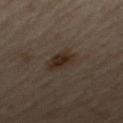<lesion>
  <biopsy_status>not biopsied; imaged during a skin examination</biopsy_status>
  <automated_metrics>
    <nevus_likeness_0_100>95</nevus_likeness_0_100>
    <lesion_detection_confidence_0_100>100</lesion_detection_confidence_0_100>
  </automated_metrics>
  <lesion_size>
    <long_diameter_mm_approx>3.5</long_diameter_mm_approx>
  </lesion_size>
  <image>
    <source>total-body photography crop</source>
    <field_of_view_mm>15</field_of_view_mm>
  </image>
  <lighting>cross-polarized</lighting>
  <patient>
    <sex>male</sex>
    <age_approx>65</age_approx>
  </patient>
  <site>leg</site>
</lesion>